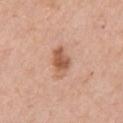Part of a total-body skin-imaging series; this lesion was reviewed on a skin check and was not flagged for biopsy. This is a white-light tile. On the right upper arm. Approximately 4 mm at its widest. A female subject, about 65 years old. A 15 mm close-up extracted from a 3D total-body photography capture.